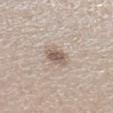notes: imaged on a skin check; not biopsied | location: the left lower leg | subject: male, roughly 50 years of age | image: ~15 mm tile from a whole-body skin photo.